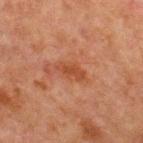notes: no biopsy performed (imaged during a skin exam) | patient: male, about 65 years old | body site: the chest | lesion diameter: about 3.5 mm | image: ~15 mm tile from a whole-body skin photo | TBP lesion metrics: a lesion color around L≈38 a*≈23 b*≈30 in CIELAB, a lesion–skin lightness drop of about 7, and a lesion-to-skin contrast of about 7 (normalized; higher = more distinct); a border-irregularity index near 3/10, a color-variation rating of about 1.5/10, and radial color variation of about 0.5; a classifier nevus-likeness of about 0/100 and a detector confidence of about 100 out of 100 that the crop contains a lesion.